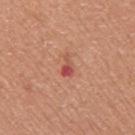The lesion was tiled from a total-body skin photograph and was not biopsied. A region of skin cropped from a whole-body photographic capture, roughly 15 mm wide. A female patient about 60 years old. The lesion is located on the right upper arm. Imaged with white-light lighting. About 2.5 mm across. Automated tile analysis of the lesion measured a mean CIELAB color near L≈52 a*≈32 b*≈30, about 11 CIELAB-L* units darker than the surrounding skin, and a normalized border contrast of about 7.5. And it measured a border-irregularity rating of about 5/10, a color-variation rating of about 1.5/10, and a peripheral color-asymmetry measure near 0.5. The analysis additionally found a nevus-likeness score of about 0/100 and lesion-presence confidence of about 100/100.